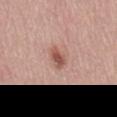<lesion>
<biopsy_status>not biopsied; imaged during a skin examination</biopsy_status>
<patient>
  <sex>male</sex>
  <age_approx>60</age_approx>
</patient>
<site>mid back</site>
<image>
  <source>total-body photography crop</source>
  <field_of_view_mm>15</field_of_view_mm>
</image>
</lesion>